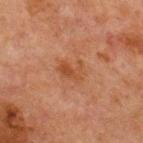Case summary:
- follow-up: imaged on a skin check; not biopsied
- automated lesion analysis: a footprint of about 5 mm², a shape eccentricity near 0.75, and two-axis asymmetry of about 0.4; about 6 CIELAB-L* units darker than the surrounding skin; a classifier nevus-likeness of about 0/100 and a lesion-detection confidence of about 100/100
- lesion diameter: about 3 mm
- lighting: cross-polarized illumination
- patient: male, aged around 65
- location: the chest
- image source: 15 mm crop, total-body photography